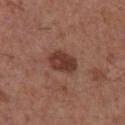Impression: No biopsy was performed on this lesion — it was imaged during a full skin examination and was not determined to be concerning. Background: Cropped from a whole-body photographic skin survey; the tile spans about 15 mm. A male patient, roughly 55 years of age. From the front of the torso. This is a white-light tile. Approximately 4 mm at its widest. The total-body-photography lesion software estimated a symmetry-axis asymmetry near 0.15. And it measured a lesion color around L≈38 a*≈23 b*≈25 in CIELAB, a lesion–skin lightness drop of about 12, and a normalized border contrast of about 9.5.